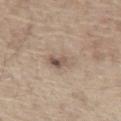The lesion was tiled from a total-body skin photograph and was not biopsied. A male subject in their mid- to late 60s. Automated image analysis of the tile measured a footprint of about 11 mm² and an outline eccentricity of about 0.6 (0 = round, 1 = elongated). It also reported a lesion color around L≈58 a*≈12 b*≈25 in CIELAB, roughly 8 lightness units darker than nearby skin, and a normalized border contrast of about 5. The software also gave a border-irregularity index near 2.5/10 and internal color variation of about 8.5 on a 0–10 scale. Cropped from a total-body skin-imaging series; the visible field is about 15 mm. The lesion is located on the chest. Imaged with white-light lighting. Longest diameter approximately 4 mm.